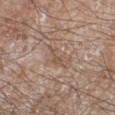Impression: Imaged during a routine full-body skin examination; the lesion was not biopsied and no histopathology is available. Background: A male subject approximately 60 years of age. The lesion is on the right lower leg. This image is a 15 mm lesion crop taken from a total-body photograph.